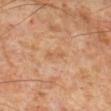biopsy status = no biopsy performed (imaged during a skin exam)
subject = male, about 60 years old
acquisition = total-body-photography crop, ~15 mm field of view
site = the leg
lesion size = ~2.5 mm (longest diameter)